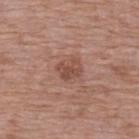biopsy status: total-body-photography surveillance lesion; no biopsy | anatomic site: the upper back | subject: female, aged approximately 65 | lighting: white-light | lesion diameter: ≈3 mm | image source: ~15 mm tile from a whole-body skin photo.